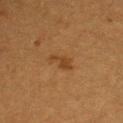• image source · ~15 mm crop, total-body skin-cancer survey
• lighting · cross-polarized
• size · ≈4 mm
• anatomic site · the chest
• automated metrics · a lesion area of about 4.5 mm², an outline eccentricity of about 0.9 (0 = round, 1 = elongated), and two-axis asymmetry of about 0.3; a detector confidence of about 100 out of 100 that the crop contains a lesion
• subject · female, aged 53–57